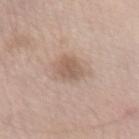Impression: This lesion was catalogued during total-body skin photography and was not selected for biopsy. Background: The tile uses white-light illumination. A female subject, roughly 55 years of age. About 3 mm across. This image is a 15 mm lesion crop taken from a total-body photograph. On the front of the torso.